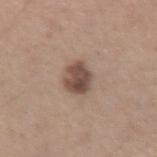workup = catalogued during a skin exam; not biopsied
location = the left upper arm
image = 15 mm crop, total-body photography
subject = male, aged around 45
illumination = white-light illumination